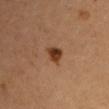{
  "biopsy_status": "not biopsied; imaged during a skin examination",
  "site": "left upper arm",
  "image": {
    "source": "total-body photography crop",
    "field_of_view_mm": 15
  },
  "patient": {
    "sex": "female",
    "age_approx": 35
  }
}